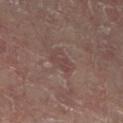Assessment:
The lesion was photographed on a routine skin check and not biopsied; there is no pathology result.
Acquisition and patient details:
The lesion's longest dimension is about 2.5 mm. A close-up tile cropped from a whole-body skin photograph, about 15 mm across. Automated image analysis of the tile measured a footprint of about 3.5 mm², a shape eccentricity near 0.85, and a symmetry-axis asymmetry near 0.35. It also reported a border-irregularity index near 4/10, a color-variation rating of about 0.5/10, and radial color variation of about 0. The analysis additionally found a nevus-likeness score of about 0/100. A male subject, aged 58–62. The lesion is on the left lower leg.